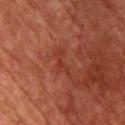{"biopsy_status": "not biopsied; imaged during a skin examination", "image": {"source": "total-body photography crop", "field_of_view_mm": 15}, "automated_metrics": {"cielab_L": 37, "cielab_a": 30, "cielab_b": 32, "vs_skin_darker_L": 5.0, "vs_skin_contrast_norm": 5.0}, "patient": {"sex": "male", "age_approx": 70}, "site": "chest"}